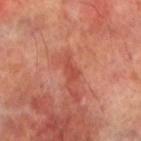The lesion-visualizer software estimated a lesion area of about 4 mm², an eccentricity of roughly 0.9, and a shape-asymmetry score of about 0.35 (0 = symmetric). The software also gave a mean CIELAB color near L≈49 a*≈32 b*≈32, about 8 CIELAB-L* units darker than the surrounding skin, and a normalized border contrast of about 6. The lesion is on the left lower leg. A male subject, aged approximately 70. A roughly 15 mm field-of-view crop from a total-body skin photograph. Captured under cross-polarized illumination. Measured at roughly 3 mm in maximum diameter.